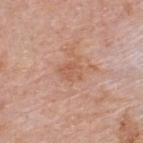The lesion was tiled from a total-body skin photograph and was not biopsied. A 15 mm close-up extracted from a 3D total-body photography capture. A male subject about 75 years old. The recorded lesion diameter is about 2.5 mm. On the back. Imaged with white-light lighting.